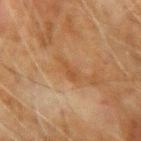Case summary:
- patient · male, aged around 70
- acquisition · total-body-photography crop, ~15 mm field of view
- site · the left upper arm